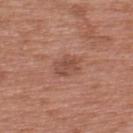notes — no biopsy performed (imaged during a skin exam)
illumination — white-light illumination
lesion size — ≈3 mm
site — the upper back
patient — male, aged around 70
image source — 15 mm crop, total-body photography